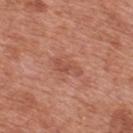Q: Was a biopsy performed?
A: imaged on a skin check; not biopsied
Q: Patient demographics?
A: male, in their 70s
Q: What is the imaging modality?
A: total-body-photography crop, ~15 mm field of view
Q: Automated lesion metrics?
A: a lesion area of about 5 mm², an outline eccentricity of about 0.85 (0 = round, 1 = elongated), and two-axis asymmetry of about 0.4; border irregularity of about 4.5 on a 0–10 scale and peripheral color asymmetry of about 0.5
Q: How was the tile lit?
A: white-light
Q: What is the anatomic site?
A: the upper back
Q: How large is the lesion?
A: ≈3.5 mm